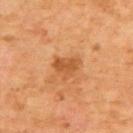Assessment: No biopsy was performed on this lesion — it was imaged during a full skin examination and was not determined to be concerning. Image and clinical context: Longest diameter approximately 3.5 mm. The lesion is on the upper back. A male subject roughly 65 years of age. A region of skin cropped from a whole-body photographic capture, roughly 15 mm wide. Captured under cross-polarized illumination.